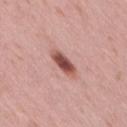The lesion is located on the right thigh.
A female subject in their 40s.
A lesion tile, about 15 mm wide, cut from a 3D total-body photograph.